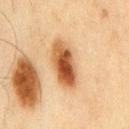<case>
  <biopsy_status>not biopsied; imaged during a skin examination</biopsy_status>
  <patient>
    <sex>male</sex>
    <age_approx>45</age_approx>
  </patient>
  <image>
    <source>total-body photography crop</source>
    <field_of_view_mm>15</field_of_view_mm>
  </image>
  <site>front of the torso</site>
  <lighting>cross-polarized</lighting>
  <automated_metrics>
    <eccentricity>0.9</eccentricity>
    <shape_asymmetry>0.15</shape_asymmetry>
    <vs_skin_darker_L>15.0</vs_skin_darker_L>
    <vs_skin_contrast_norm>11.5</vs_skin_contrast_norm>
    <lesion_detection_confidence_0_100>95</lesion_detection_confidence_0_100>
  </automated_metrics>
</case>